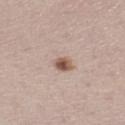| field | value |
|---|---|
| workup | catalogued during a skin exam; not biopsied |
| location | the right lower leg |
| tile lighting | white-light |
| lesion size | ~4 mm (longest diameter) |
| patient | female, aged 38–42 |
| imaging modality | total-body-photography crop, ~15 mm field of view |